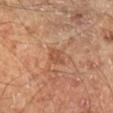The lesion was tiled from a total-body skin photograph and was not biopsied.
Approximately 2 mm at its widest.
Automated tile analysis of the lesion measured lesion-presence confidence of about 100/100.
Cropped from a whole-body photographic skin survey; the tile spans about 15 mm.
The lesion is located on the leg.
The tile uses cross-polarized illumination.
A male subject, in their 60s.